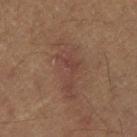<record>
  <image>
    <source>total-body photography crop</source>
    <field_of_view_mm>15</field_of_view_mm>
  </image>
  <lesion_size>
    <long_diameter_mm_approx>7.5</long_diameter_mm_approx>
  </lesion_size>
  <patient>
    <sex>male</sex>
    <age_approx>60</age_approx>
  </patient>
</record>